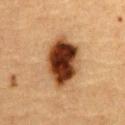• biopsy status — catalogued during a skin exam; not biopsied
• image — ~15 mm tile from a whole-body skin photo
• automated lesion analysis — an average lesion color of about L≈35 a*≈21 b*≈31 (CIELAB), about 22 CIELAB-L* units darker than the surrounding skin, and a lesion-to-skin contrast of about 17 (normalized; higher = more distinct); a border-irregularity index near 1.5/10 and a within-lesion color-variation index near 9.5/10; an automated nevus-likeness rating near 100 out of 100
• location — the abdomen
• subject — male, aged around 85 or older
• lesion size — ~6.5 mm (longest diameter)
• lighting — cross-polarized